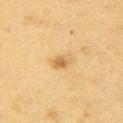image:
  source: total-body photography crop
  field_of_view_mm: 15
patient:
  sex: male
  age_approx: 60
site: right upper arm